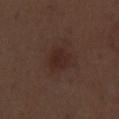Captured during whole-body skin photography for melanoma surveillance; the lesion was not biopsied.
The lesion-visualizer software estimated a lesion area of about 6 mm², an eccentricity of roughly 0.45, and two-axis asymmetry of about 0.25. It also reported border irregularity of about 2 on a 0–10 scale, a within-lesion color-variation index near 2/10, and radial color variation of about 1.
Imaged with white-light lighting.
A male patient, aged approximately 70.
A 15 mm close-up tile from a total-body photography series done for melanoma screening.
From the left thigh.
The lesion's longest dimension is about 3 mm.